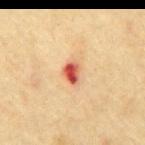• follow-up · imaged on a skin check; not biopsied
• size · ~2.5 mm (longest diameter)
• subject · male, aged 68–72
• illumination · cross-polarized
• location · the mid back
• automated lesion analysis · an area of roughly 4.5 mm², an outline eccentricity of about 0.7 (0 = round, 1 = elongated), and a shape-asymmetry score of about 0.25 (0 = symmetric); a mean CIELAB color near L≈56 a*≈33 b*≈35 and roughly 17 lightness units darker than nearby skin; a within-lesion color-variation index near 9/10; a nevus-likeness score of about 0/100 and a lesion-detection confidence of about 100/100
• acquisition · ~15 mm tile from a whole-body skin photo